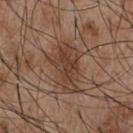The lesion was photographed on a routine skin check and not biopsied; there is no pathology result.
A male patient in their mid- to late 50s.
A close-up tile cropped from a whole-body skin photograph, about 15 mm across.
On the chest.
Imaged with white-light lighting.
Approximately 6.5 mm at its widest.